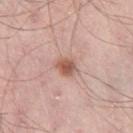Impression: The lesion was tiled from a total-body skin photograph and was not biopsied. Acquisition and patient details: A 15 mm crop from a total-body photograph taken for skin-cancer surveillance. On the right thigh. The subject is a male in their mid-50s. Approximately 2.5 mm at its widest.